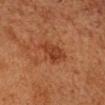subject — male, approximately 65 years of age | image — ~15 mm crop, total-body skin-cancer survey | site — the head or neck | lighting — cross-polarized illumination.